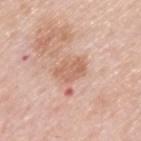The lesion was photographed on a routine skin check and not biopsied; there is no pathology result. From the upper back. Cropped from a total-body skin-imaging series; the visible field is about 15 mm. A male patient, aged approximately 60.